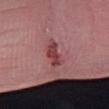Imaged during a routine full-body skin examination; the lesion was not biopsied and no histopathology is available. This image is a 15 mm lesion crop taken from a total-body photograph. Captured under white-light illumination. Longest diameter approximately 4.5 mm. A male patient, approximately 55 years of age.